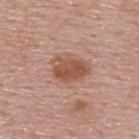The lesion is located on the upper back.
A male patient approximately 55 years of age.
Cropped from a whole-body photographic skin survey; the tile spans about 15 mm.